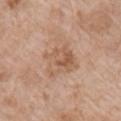Assessment:
Captured during whole-body skin photography for melanoma surveillance; the lesion was not biopsied.
Background:
This is a white-light tile. A female patient aged approximately 75. The lesion is on the right upper arm. A roughly 15 mm field-of-view crop from a total-body skin photograph. Automated tile analysis of the lesion measured a nevus-likeness score of about 0/100 and a detector confidence of about 100 out of 100 that the crop contains a lesion.